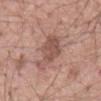Part of a total-body skin-imaging series; this lesion was reviewed on a skin check and was not flagged for biopsy. A male patient about 55 years old. A 15 mm crop from a total-body photograph taken for skin-cancer surveillance. On the mid back.